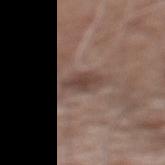| key | value |
|---|---|
| follow-up | no biopsy performed (imaged during a skin exam) |
| size | ~4 mm (longest diameter) |
| TBP lesion metrics | a lesion color around L≈43 a*≈16 b*≈22 in CIELAB, a lesion–skin lightness drop of about 9, and a normalized lesion–skin contrast near 7.5 |
| acquisition | ~15 mm crop, total-body skin-cancer survey |
| patient | male, in their 60s |
| tile lighting | white-light |
| body site | the mid back |